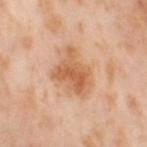Assessment: The lesion was tiled from a total-body skin photograph and was not biopsied. Background: A 15 mm close-up extracted from a 3D total-body photography capture. The lesion is located on the leg. A female patient aged 53 to 57.